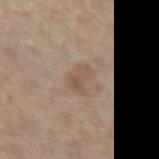<record>
<biopsy_status>not biopsied; imaged during a skin examination</biopsy_status>
<patient>
  <sex>male</sex>
  <age_approx>70</age_approx>
</patient>
<lighting>white-light</lighting>
<site>left forearm</site>
<image>
  <source>total-body photography crop</source>
  <field_of_view_mm>15</field_of_view_mm>
</image>
<lesion_size>
  <long_diameter_mm_approx>3.0</long_diameter_mm_approx>
</lesion_size>
</record>